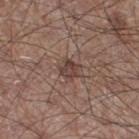  biopsy_status: not biopsied; imaged during a skin examination
  patient:
    sex: male
    age_approx: 60
  lighting: white-light
  site: right thigh
  image:
    source: total-body photography crop
    field_of_view_mm: 15
  lesion_size:
    long_diameter_mm_approx: 3.0